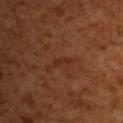Context: The patient is a female approximately 55 years of age. This image is a 15 mm lesion crop taken from a total-body photograph. The lesion is on the upper back.